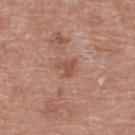Context:
The tile uses white-light illumination. From the back. Longest diameter approximately 2.5 mm. A roughly 15 mm field-of-view crop from a total-body skin photograph. A female patient in their 60s.